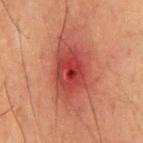Q: Was a biopsy performed?
A: no biopsy performed (imaged during a skin exam)
Q: Lesion size?
A: about 8.5 mm
Q: How was this image acquired?
A: ~15 mm crop, total-body skin-cancer survey
Q: What did automated image analysis measure?
A: an area of roughly 29 mm², an eccentricity of roughly 0.8, and a symmetry-axis asymmetry near 0.2; a within-lesion color-variation index near 6/10 and peripheral color asymmetry of about 1.5; an automated nevus-likeness rating near 0 out of 100 and lesion-presence confidence of about 100/100
Q: Illumination type?
A: cross-polarized illumination
Q: What is the anatomic site?
A: the chest
Q: What are the patient's age and sex?
A: male, aged 48 to 52